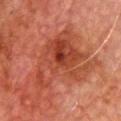image:
  source: total-body photography crop
  field_of_view_mm: 15
patient:
  sex: male
  age_approx: 75
site: chest